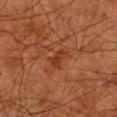workup: imaged on a skin check; not biopsied | acquisition: 15 mm crop, total-body photography | anatomic site: the left lower leg | subject: male, aged 78 to 82 | illumination: cross-polarized | lesion size: about 2.5 mm.